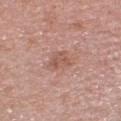The lesion was tiled from a total-body skin photograph and was not biopsied. A female patient, aged approximately 60. Cropped from a total-body skin-imaging series; the visible field is about 15 mm. About 2.5 mm across. On the head or neck. The total-body-photography lesion software estimated a lesion area of about 4 mm² and two-axis asymmetry of about 0.35. The analysis additionally found a lesion color around L≈55 a*≈23 b*≈27 in CIELAB, roughly 8 lightness units darker than nearby skin, and a normalized border contrast of about 6.